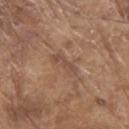Assessment: No biopsy was performed on this lesion — it was imaged during a full skin examination and was not determined to be concerning. Background: On the arm. Longest diameter approximately 3.5 mm. Automated tile analysis of the lesion measured a footprint of about 5 mm², a shape eccentricity near 0.9, and two-axis asymmetry of about 0.3. The software also gave an average lesion color of about L≈49 a*≈18 b*≈27 (CIELAB) and a lesion-to-skin contrast of about 5.5 (normalized; higher = more distinct). The analysis additionally found border irregularity of about 4 on a 0–10 scale and peripheral color asymmetry of about 0.5. A 15 mm crop from a total-body photograph taken for skin-cancer surveillance. The subject is a male about 80 years old. Captured under white-light illumination.